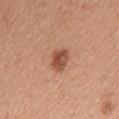Impression:
This lesion was catalogued during total-body skin photography and was not selected for biopsy.
Background:
The subject is a female aged 43–47. The lesion is on the upper back. Cropped from a total-body skin-imaging series; the visible field is about 15 mm.